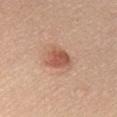This lesion was catalogued during total-body skin photography and was not selected for biopsy.
This is a white-light tile.
The patient is a female aged 53–57.
Cropped from a total-body skin-imaging series; the visible field is about 15 mm.
Longest diameter approximately 3.5 mm.
The lesion is on the chest.